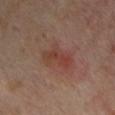Case summary:
* workup · catalogued during a skin exam; not biopsied
* imaging modality · total-body-photography crop, ~15 mm field of view
* site · the chest
* subject · male, roughly 45 years of age
* lesion diameter · about 5 mm
* automated metrics · an area of roughly 10 mm², an eccentricity of roughly 0.85, and a symmetry-axis asymmetry near 0.3; about 6 CIELAB-L* units darker than the surrounding skin and a lesion-to-skin contrast of about 6 (normalized; higher = more distinct); a classifier nevus-likeness of about 90/100 and a lesion-detection confidence of about 100/100